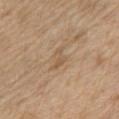The lesion was photographed on a routine skin check and not biopsied; there is no pathology result. A male subject aged 68–72. Imaged with white-light lighting. The lesion is on the left upper arm. This image is a 15 mm lesion crop taken from a total-body photograph. Measured at roughly 2.5 mm in maximum diameter. Automated image analysis of the tile measured an average lesion color of about L≈55 a*≈16 b*≈33 (CIELAB), a lesion–skin lightness drop of about 7, and a lesion-to-skin contrast of about 5 (normalized; higher = more distinct). And it measured a border-irregularity index near 7/10, a color-variation rating of about 0/10, and peripheral color asymmetry of about 0.